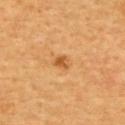The lesion was photographed on a routine skin check and not biopsied; there is no pathology result. A 15 mm close-up tile from a total-body photography series done for melanoma screening. On the upper back. A female patient aged around 50.